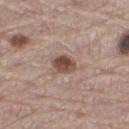| feature | finding |
|---|---|
| imaging modality | 15 mm crop, total-body photography |
| site | the right thigh |
| size | about 3 mm |
| subject | male, in their mid-60s |